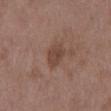Findings:
* imaging modality · ~15 mm crop, total-body skin-cancer survey
* body site · the mid back
* subject · male, roughly 50 years of age